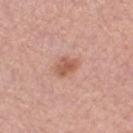The lesion was tiled from a total-body skin photograph and was not biopsied. The lesion is on the right thigh. A 15 mm crop from a total-body photograph taken for skin-cancer surveillance. A female patient aged 68–72.